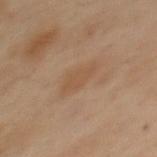Assessment: This lesion was catalogued during total-body skin photography and was not selected for biopsy. Context: The tile uses cross-polarized illumination. The recorded lesion diameter is about 3.5 mm. Located on the upper back. A female patient about 55 years old. A roughly 15 mm field-of-view crop from a total-body skin photograph.